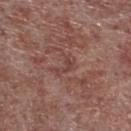The lesion was tiled from a total-body skin photograph and was not biopsied. Cropped from a whole-body photographic skin survey; the tile spans about 15 mm. Automated image analysis of the tile measured a lesion–skin lightness drop of about 6 and a normalized lesion–skin contrast near 5. And it measured a classifier nevus-likeness of about 0/100 and a detector confidence of about 70 out of 100 that the crop contains a lesion. The lesion is located on the leg. The patient is a male aged 53 to 57.